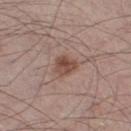Recorded during total-body skin imaging; not selected for excision or biopsy.
A lesion tile, about 15 mm wide, cut from a 3D total-body photograph.
On the right thigh.
This is a white-light tile.
The subject is a male aged 58 to 62.